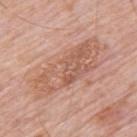{"biopsy_status": "not biopsied; imaged during a skin examination", "image": {"source": "total-body photography crop", "field_of_view_mm": 15}, "lesion_size": {"long_diameter_mm_approx": 9.5}, "site": "upper back", "patient": {"sex": "male", "age_approx": 80}}